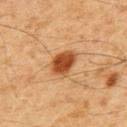biopsy status=no biopsy performed (imaged during a skin exam); location=the upper back; image source=15 mm crop, total-body photography; subject=male, approximately 60 years of age; lesion diameter=~3.5 mm (longest diameter).